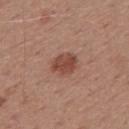Clinical impression: Recorded during total-body skin imaging; not selected for excision or biopsy. Acquisition and patient details: Cropped from a total-body skin-imaging series; the visible field is about 15 mm. About 3 mm across. A male patient aged 53–57. The tile uses white-light illumination. Located on the mid back.